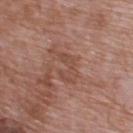Imaged during a routine full-body skin examination; the lesion was not biopsied and no histopathology is available. The subject is a male aged 68–72. The lesion is located on the upper back. A 15 mm close-up extracted from a 3D total-body photography capture.